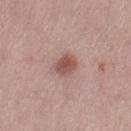biopsy status = no biopsy performed (imaged during a skin exam); anatomic site = the left thigh; patient = female, approximately 60 years of age; imaging modality = 15 mm crop, total-body photography; tile lighting = white-light.